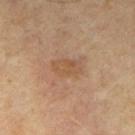This lesion was catalogued during total-body skin photography and was not selected for biopsy.
The patient is a male aged around 65.
The lesion-visualizer software estimated an eccentricity of roughly 0.8 and two-axis asymmetry of about 0.2. It also reported a lesion color around L≈51 a*≈17 b*≈32 in CIELAB, a lesion–skin lightness drop of about 6, and a lesion-to-skin contrast of about 5 (normalized; higher = more distinct). And it measured border irregularity of about 2.5 on a 0–10 scale. The analysis additionally found an automated nevus-likeness rating near 5 out of 100 and lesion-presence confidence of about 100/100.
A 15 mm close-up extracted from a 3D total-body photography capture.
About 3.5 mm across.